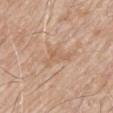No biopsy was performed on this lesion — it was imaged during a full skin examination and was not determined to be concerning.
The patient is a male aged approximately 80.
A close-up tile cropped from a whole-body skin photograph, about 15 mm across.
The lesion is located on the mid back.